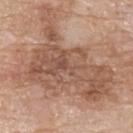Q: What did automated image analysis measure?
A: an outline eccentricity of about 0.75 (0 = round, 1 = elongated) and a symmetry-axis asymmetry near 0.55; a border-irregularity index near 9/10, a within-lesion color-variation index near 5/10, and radial color variation of about 2; an automated nevus-likeness rating near 0 out of 100 and a detector confidence of about 100 out of 100 that the crop contains a lesion
Q: What is the imaging modality?
A: ~15 mm crop, total-body skin-cancer survey
Q: What are the patient's age and sex?
A: male, aged 78–82
Q: What is the anatomic site?
A: the upper back
Q: How large is the lesion?
A: about 13 mm